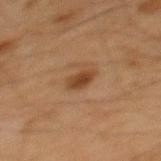biopsy_status: not biopsied; imaged during a skin examination
lighting: cross-polarized
patient:
  sex: male
  age_approx: 65
lesion_size:
  long_diameter_mm_approx: 2.5
automated_metrics:
  area_mm2_approx: 3.0
  eccentricity: 0.8
  cielab_L: 34
  cielab_a: 19
  cielab_b: 30
  vs_skin_darker_L: 10.0
  nevus_likeness_0_100: 90
  lesion_detection_confidence_0_100: 100
image:
  source: total-body photography crop
  field_of_view_mm: 15
site: mid back